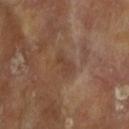Automated image analysis of the tile measured two-axis asymmetry of about 0.3.
Captured under cross-polarized illumination.
Longest diameter approximately 3.5 mm.
The lesion is located on the left forearm.
A female patient, in their mid-70s.
A region of skin cropped from a whole-body photographic capture, roughly 15 mm wide.